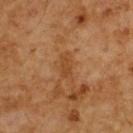Captured during whole-body skin photography for melanoma surveillance; the lesion was not biopsied. The tile uses cross-polarized illumination. The lesion is on the upper back. A close-up tile cropped from a whole-body skin photograph, about 15 mm across. The subject is a male approximately 60 years of age.